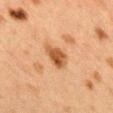  image:
    source: total-body photography crop
    field_of_view_mm: 15
  automated_metrics:
    cielab_L: 46
    cielab_a: 21
    cielab_b: 35
    vs_skin_darker_L: 11.0
    vs_skin_contrast_norm: 9.0
    border_irregularity_0_10: 3.0
    peripheral_color_asymmetry: 1.0
    lesion_detection_confidence_0_100: 100
  site: upper back
  patient:
    sex: female
    age_approx: 40
  lesion_size:
    long_diameter_mm_approx: 4.0
  lighting: cross-polarized